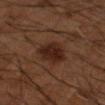This lesion was catalogued during total-body skin photography and was not selected for biopsy. Measured at roughly 4.5 mm in maximum diameter. Located on the arm. Automated image analysis of the tile measured a lesion area of about 10 mm². A region of skin cropped from a whole-body photographic capture, roughly 15 mm wide. A male patient, aged approximately 55.